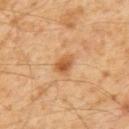This lesion was catalogued during total-body skin photography and was not selected for biopsy. The patient is a male roughly 60 years of age. A close-up tile cropped from a whole-body skin photograph, about 15 mm across. This is a cross-polarized tile. The lesion is on the mid back.